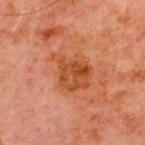Case summary:
- workup: no biopsy performed (imaged during a skin exam)
- image: 15 mm crop, total-body photography
- subject: male, in their 70s
- anatomic site: the chest